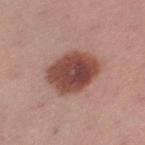Impression:
Imaged during a routine full-body skin examination; the lesion was not biopsied and no histopathology is available.
Background:
From the left thigh. A female patient in their mid-50s. Captured under white-light illumination. Measured at roughly 5.5 mm in maximum diameter. A region of skin cropped from a whole-body photographic capture, roughly 15 mm wide.